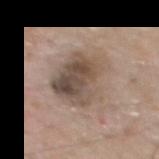follow-up — no biopsy performed (imaged during a skin exam)
size — ~7 mm (longest diameter)
image source — total-body-photography crop, ~15 mm field of view
location — the mid back
patient — male, aged 58 to 62